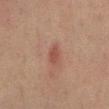Impression: Recorded during total-body skin imaging; not selected for excision or biopsy. Acquisition and patient details: The lesion is on the mid back. The subject is a male in their 70s. Approximately 3 mm at its widest. Automated tile analysis of the lesion measured a border-irregularity rating of about 2.5/10 and a peripheral color-asymmetry measure near 0. The tile uses cross-polarized illumination. A lesion tile, about 15 mm wide, cut from a 3D total-body photograph.